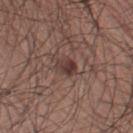• illumination · white-light
• lesion size · ~3 mm (longest diameter)
• body site · the left thigh
• subject · male, aged 28 to 32
• image · ~15 mm crop, total-body skin-cancer survey